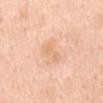image-analysis metrics = roughly 6 lightness units darker than nearby skin and a normalized lesion–skin contrast near 4.5
subject = male, approximately 60 years of age
location = the mid back
image = ~15 mm tile from a whole-body skin photo
tile lighting = white-light illumination